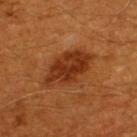The lesion was photographed on a routine skin check and not biopsied; there is no pathology result.
Located on the upper back.
A roughly 15 mm field-of-view crop from a total-body skin photograph.
A male patient in their 60s.